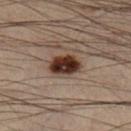The lesion was photographed on a routine skin check and not biopsied; there is no pathology result.
A lesion tile, about 15 mm wide, cut from a 3D total-body photograph.
On the right lower leg.
A male patient about 55 years old.